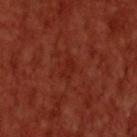  biopsy_status: not biopsied; imaged during a skin examination
  lighting: cross-polarized
  image:
    source: total-body photography crop
    field_of_view_mm: 15
  automated_metrics:
    area_mm2_approx: 3.0
    eccentricity: 0.85
    shape_asymmetry: 0.4
    vs_skin_contrast_norm: 4.5
    border_irregularity_0_10: 3.5
    color_variation_0_10: 0.5
    peripheral_color_asymmetry: 0.0
    nevus_likeness_0_100: 0
    lesion_detection_confidence_0_100: 100
  site: head or neck
  patient:
    sex: male
    age_approx: 60
  lesion_size:
    long_diameter_mm_approx: 2.5